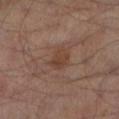Notes:
– image-analysis metrics — a lesion color around L≈38 a*≈18 b*≈26 in CIELAB and roughly 6 lightness units darker than nearby skin; a border-irregularity index near 2/10 and internal color variation of about 2.5 on a 0–10 scale; a nevus-likeness score of about 20/100 and a lesion-detection confidence of about 100/100
– size — ~3 mm (longest diameter)
– imaging modality — ~15 mm crop, total-body skin-cancer survey
– illumination — cross-polarized illumination
– subject — male, aged approximately 65
– site — the leg